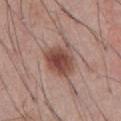The lesion was photographed on a routine skin check and not biopsied; there is no pathology result.
On the abdomen.
Imaged with white-light lighting.
A 15 mm crop from a total-body photograph taken for skin-cancer surveillance.
The recorded lesion diameter is about 4 mm.
The subject is a male in their mid- to late 50s.
Automated tile analysis of the lesion measured a border-irregularity rating of about 3/10 and a within-lesion color-variation index near 5/10.